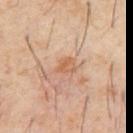Part of a total-body skin-imaging series; this lesion was reviewed on a skin check and was not flagged for biopsy.
A male patient aged approximately 60.
A close-up tile cropped from a whole-body skin photograph, about 15 mm across.
Automated image analysis of the tile measured a lesion color around L≈56 a*≈20 b*≈32 in CIELAB, roughly 7 lightness units darker than nearby skin, and a lesion-to-skin contrast of about 6 (normalized; higher = more distinct). The software also gave a color-variation rating of about 2.5/10 and peripheral color asymmetry of about 1. And it measured an automated nevus-likeness rating near 15 out of 100 and a lesion-detection confidence of about 100/100.
Captured under cross-polarized illumination.
On the abdomen.
Measured at roughly 2.5 mm in maximum diameter.